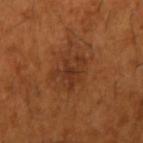No biopsy was performed on this lesion — it was imaged during a full skin examination and was not determined to be concerning. The lesion-visualizer software estimated an average lesion color of about L≈34 a*≈23 b*≈33 (CIELAB), a lesion–skin lightness drop of about 7, and a lesion-to-skin contrast of about 6.5 (normalized; higher = more distinct). The analysis additionally found a color-variation rating of about 3/10. The analysis additionally found a nevus-likeness score of about 0/100 and lesion-presence confidence of about 100/100. A male subject, in their mid-60s. The lesion is located on the arm. The recorded lesion diameter is about 4 mm. The tile uses cross-polarized illumination. A close-up tile cropped from a whole-body skin photograph, about 15 mm across.